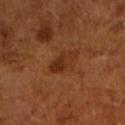Captured during whole-body skin photography for melanoma surveillance; the lesion was not biopsied. The patient is a male aged approximately 65. A 15 mm close-up extracted from a 3D total-body photography capture. Imaged with cross-polarized lighting.